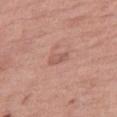| key | value |
|---|---|
| biopsy status | catalogued during a skin exam; not biopsied |
| site | the right thigh |
| image source | 15 mm crop, total-body photography |
| patient | female, about 70 years old |